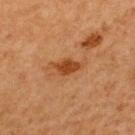  biopsy_status: not biopsied; imaged during a skin examination
  site: back
  image:
    source: total-body photography crop
    field_of_view_mm: 15
  patient:
    sex: male
    age_approx: 65
  lesion_size:
    long_diameter_mm_approx: 3.0